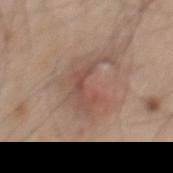The lesion was photographed on a routine skin check and not biopsied; there is no pathology result. A male subject, in their mid-40s. The lesion's longest dimension is about 5.5 mm. This image is a 15 mm lesion crop taken from a total-body photograph. From the mid back. The lesion-visualizer software estimated a symmetry-axis asymmetry near 0.6. The software also gave a classifier nevus-likeness of about 15/100 and a detector confidence of about 100 out of 100 that the crop contains a lesion. The tile uses white-light illumination.